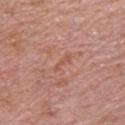A male subject, approximately 70 years of age.
This is a white-light tile.
Located on the chest.
The total-body-photography lesion software estimated an area of roughly 2 mm² and an eccentricity of roughly 0.95. And it measured a classifier nevus-likeness of about 0/100 and a lesion-detection confidence of about 95/100.
A lesion tile, about 15 mm wide, cut from a 3D total-body photograph.